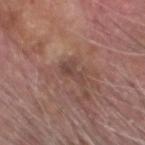<tbp_lesion>
  <biopsy_status>not biopsied; imaged during a skin examination</biopsy_status>
</tbp_lesion>